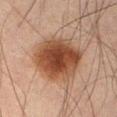biopsy status: catalogued during a skin exam; not biopsied | location: the lower back | automated metrics: an outline eccentricity of about 0.5 (0 = round, 1 = elongated) and a shape-asymmetry score of about 0.15 (0 = symmetric); a mean CIELAB color near L≈38 a*≈19 b*≈27 and about 13 CIELAB-L* units darker than the surrounding skin | image: total-body-photography crop, ~15 mm field of view | lighting: cross-polarized | patient: male, roughly 70 years of age.